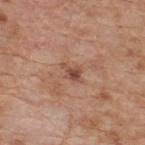Clinical impression: The lesion was photographed on a routine skin check and not biopsied; there is no pathology result.